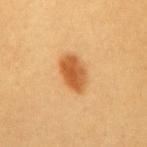• follow-up — imaged on a skin check; not biopsied